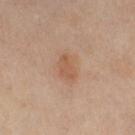The lesion was tiled from a total-body skin photograph and was not biopsied.